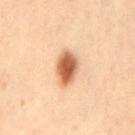The lesion was tiled from a total-body skin photograph and was not biopsied.
A female subject, aged around 40.
The total-body-photography lesion software estimated a footprint of about 8.5 mm², an eccentricity of roughly 0.8, and two-axis asymmetry of about 0.15. And it measured an average lesion color of about L≈64 a*≈26 b*≈39 (CIELAB) and about 20 CIELAB-L* units darker than the surrounding skin.
Approximately 4.5 mm at its widest.
On the right leg.
Cropped from a total-body skin-imaging series; the visible field is about 15 mm.